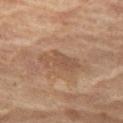Impression:
The lesion was tiled from a total-body skin photograph and was not biopsied.
Acquisition and patient details:
A female patient in their 70s. The total-body-photography lesion software estimated a nevus-likeness score of about 0/100 and lesion-presence confidence of about 85/100. Imaged with cross-polarized lighting. The lesion's longest dimension is about 5 mm. The lesion is on the left thigh. A 15 mm close-up extracted from a 3D total-body photography capture.